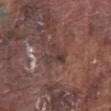This lesion was catalogued during total-body skin photography and was not selected for biopsy. The lesion is on the abdomen. A 15 mm crop from a total-body photograph taken for skin-cancer surveillance. The subject is a male in their mid-70s.